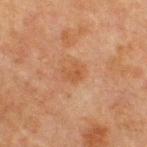Part of a total-body skin-imaging series; this lesion was reviewed on a skin check and was not flagged for biopsy. A roughly 15 mm field-of-view crop from a total-body skin photograph. Located on the chest. About 2.5 mm across. Automated image analysis of the tile measured a footprint of about 4 mm², a shape eccentricity near 0.65, and two-axis asymmetry of about 0.3. The analysis additionally found a border-irregularity index near 3/10, a color-variation rating of about 2/10, and a peripheral color-asymmetry measure near 0.5. A male subject, approximately 65 years of age.